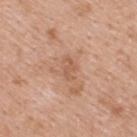workup: no biopsy performed (imaged during a skin exam); subject: female, about 40 years old; location: the back; image: 15 mm crop, total-body photography.